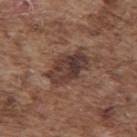Recorded during total-body skin imaging; not selected for excision or biopsy. This is a white-light tile. Located on the back. A 15 mm close-up extracted from a 3D total-body photography capture. A male subject aged 73–77. The lesion-visualizer software estimated an eccentricity of roughly 0.8 and two-axis asymmetry of about 0.2. The analysis additionally found a mean CIELAB color near L≈37 a*≈17 b*≈22, a lesion–skin lightness drop of about 11, and a normalized border contrast of about 10. The analysis additionally found internal color variation of about 6.5 on a 0–10 scale. And it measured an automated nevus-likeness rating near 5 out of 100 and a detector confidence of about 100 out of 100 that the crop contains a lesion.